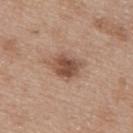biopsy_status: not biopsied; imaged during a skin examination
image:
  source: total-body photography crop
  field_of_view_mm: 15
lesion_size:
  long_diameter_mm_approx: 4.5
site: upper back
patient:
  sex: female
  age_approx: 40
automated_metrics:
  area_mm2_approx: 9.5
  eccentricity: 0.75
  shape_asymmetry: 0.3
  border_irregularity_0_10: 3.0
  color_variation_0_10: 5.0
  peripheral_color_asymmetry: 1.5
  lesion_detection_confidence_0_100: 100